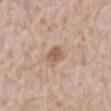| field | value |
|---|---|
| notes | imaged on a skin check; not biopsied |
| anatomic site | the abdomen |
| acquisition | ~15 mm tile from a whole-body skin photo |
| patient | male, in their 80s |
| TBP lesion metrics | a lesion area of about 4 mm² and a shape-asymmetry score of about 0.25 (0 = symmetric); a mean CIELAB color near L≈57 a*≈19 b*≈29, a lesion–skin lightness drop of about 10, and a lesion-to-skin contrast of about 7.5 (normalized; higher = more distinct); a border-irregularity rating of about 2/10 and a peripheral color-asymmetry measure near 1; an automated nevus-likeness rating near 55 out of 100 and a lesion-detection confidence of about 100/100 |
| illumination | white-light illumination |